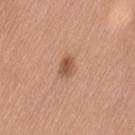Q: Was a biopsy performed?
A: total-body-photography surveillance lesion; no biopsy
Q: What is the imaging modality?
A: ~15 mm tile from a whole-body skin photo
Q: How was the tile lit?
A: white-light
Q: Who is the patient?
A: female, in their mid-50s
Q: Lesion size?
A: ~2.5 mm (longest diameter)
Q: What did automated image analysis measure?
A: an automated nevus-likeness rating near 95 out of 100 and lesion-presence confidence of about 100/100
Q: Lesion location?
A: the left thigh